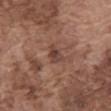From the abdomen. A close-up tile cropped from a whole-body skin photograph, about 15 mm across. A male subject aged around 75. Captured under white-light illumination.